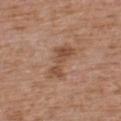notes: catalogued during a skin exam; not biopsied
anatomic site: the chest
subject: male, approximately 75 years of age
imaging modality: ~15 mm crop, total-body skin-cancer survey
size: ≈4.5 mm
image-analysis metrics: a lesion area of about 7.5 mm², a shape eccentricity near 0.9, and two-axis asymmetry of about 0.45; a mean CIELAB color near L≈49 a*≈21 b*≈30, roughly 9 lightness units darker than nearby skin, and a lesion-to-skin contrast of about 7 (normalized; higher = more distinct); a detector confidence of about 100 out of 100 that the crop contains a lesion
lighting: white-light